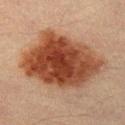Q: Patient demographics?
A: male, aged 38–42
Q: Automated lesion metrics?
A: an area of roughly 49 mm², an eccentricity of roughly 0.75, and a symmetry-axis asymmetry near 0.2; border irregularity of about 2.5 on a 0–10 scale, internal color variation of about 6 on a 0–10 scale, and peripheral color asymmetry of about 1.5
Q: How was the tile lit?
A: cross-polarized
Q: What is the imaging modality?
A: total-body-photography crop, ~15 mm field of view
Q: Where on the body is the lesion?
A: the leg
Q: Lesion size?
A: about 9.5 mm